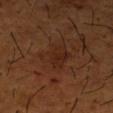workup: imaged on a skin check; not biopsied
location: the arm
illumination: cross-polarized illumination
subject: male, roughly 65 years of age
size: ~4 mm (longest diameter)
image: 15 mm crop, total-body photography